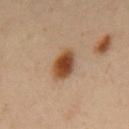Clinical impression: The lesion was photographed on a routine skin check and not biopsied; there is no pathology result. Background: Measured at roughly 4 mm in maximum diameter. Cropped from a whole-body photographic skin survey; the tile spans about 15 mm. A male patient, aged 53 to 57. The lesion is located on the back.